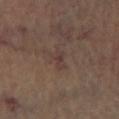The lesion was photographed on a routine skin check and not biopsied; there is no pathology result.
The lesion-visualizer software estimated an average lesion color of about L≈36 a*≈16 b*≈18 (CIELAB), a lesion–skin lightness drop of about 5, and a normalized border contrast of about 6. It also reported a border-irregularity index near 4.5/10, a within-lesion color-variation index near 1.5/10, and peripheral color asymmetry of about 0.5.
Located on the leg.
Captured under cross-polarized illumination.
The patient is aged approximately 65.
The recorded lesion diameter is about 3 mm.
This image is a 15 mm lesion crop taken from a total-body photograph.